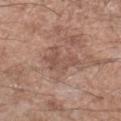biopsy status — catalogued during a skin exam; not biopsied
anatomic site — the left forearm
imaging modality — total-body-photography crop, ~15 mm field of view
tile lighting — white-light illumination
subject — male, approximately 50 years of age
size — ≈3.5 mm